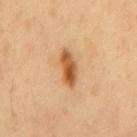Imaged during a routine full-body skin examination; the lesion was not biopsied and no histopathology is available. A 15 mm close-up tile from a total-body photography series done for melanoma screening. The patient is a male roughly 50 years of age. About 4 mm across. From the mid back.